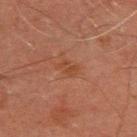The lesion was photographed on a routine skin check and not biopsied; there is no pathology result. The lesion is on the upper back. Automated tile analysis of the lesion measured a lesion color around L≈37 a*≈21 b*≈29 in CIELAB, about 5 CIELAB-L* units darker than the surrounding skin, and a normalized lesion–skin contrast near 5.5. Measured at roughly 2.5 mm in maximum diameter. Cropped from a total-body skin-imaging series; the visible field is about 15 mm. Imaged with cross-polarized lighting. A male patient aged 43 to 47.